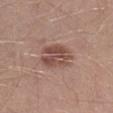Clinical impression:
Part of a total-body skin-imaging series; this lesion was reviewed on a skin check and was not flagged for biopsy.
Context:
The lesion is located on the right lower leg. The tile uses white-light illumination. A close-up tile cropped from a whole-body skin photograph, about 15 mm across. Approximately 4.5 mm at its widest. A male subject, in their 30s.